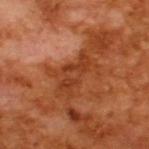{"biopsy_status": "not biopsied; imaged during a skin examination", "patient": {"sex": "male", "age_approx": 65}, "lighting": "cross-polarized", "image": {"source": "total-body photography crop", "field_of_view_mm": 15}}